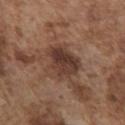Imaged during a routine full-body skin examination; the lesion was not biopsied and no histopathology is available. This is a white-light tile. A 15 mm crop from a total-body photograph taken for skin-cancer surveillance. The total-body-photography lesion software estimated an area of roughly 15 mm², a shape eccentricity near 0.6, and two-axis asymmetry of about 0.3. It also reported a mean CIELAB color near L≈38 a*≈18 b*≈25, roughly 11 lightness units darker than nearby skin, and a normalized border contrast of about 9. The analysis additionally found a within-lesion color-variation index near 6/10 and a peripheral color-asymmetry measure near 2.5. It also reported an automated nevus-likeness rating near 45 out of 100 and a detector confidence of about 100 out of 100 that the crop contains a lesion. The subject is a male aged 73 to 77. On the chest. The lesion's longest dimension is about 5 mm.